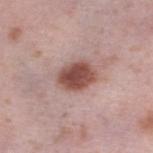<record>
  <biopsy_status>not biopsied; imaged during a skin examination</biopsy_status>
  <image>
    <source>total-body photography crop</source>
    <field_of_view_mm>15</field_of_view_mm>
  </image>
  <patient>
    <sex>female</sex>
    <age_approx>40</age_approx>
  </patient>
  <lighting>white-light</lighting>
  <site>left lower leg</site>
</record>